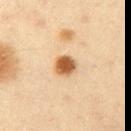• image: ~15 mm crop, total-body skin-cancer survey
• subject: male, in their mid- to late 30s
• body site: the left upper arm